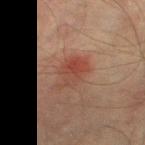Captured during whole-body skin photography for melanoma surveillance; the lesion was not biopsied. A 15 mm close-up tile from a total-body photography series done for melanoma screening. A male subject, about 75 years old. The lesion is located on the left thigh.